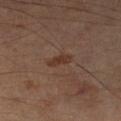| field | value |
|---|---|
| lesion diameter | ≈3 mm |
| location | the left lower leg |
| subject | male, in their mid-60s |
| image | ~15 mm crop, total-body skin-cancer survey |